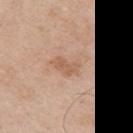Captured during whole-body skin photography for melanoma surveillance; the lesion was not biopsied. Located on the right upper arm. Captured under white-light illumination. Automated tile analysis of the lesion measured a lesion-to-skin contrast of about 6 (normalized; higher = more distinct). Longest diameter approximately 3 mm. The subject is a male aged approximately 60. A 15 mm crop from a total-body photograph taken for skin-cancer surveillance.